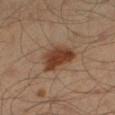Assessment: This lesion was catalogued during total-body skin photography and was not selected for biopsy. Background: The lesion is located on the left lower leg. A male patient aged approximately 40. A roughly 15 mm field-of-view crop from a total-body skin photograph.